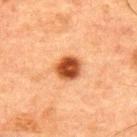notes: total-body-photography surveillance lesion; no biopsy | image source: ~15 mm tile from a whole-body skin photo | illumination: cross-polarized | lesion diameter: ~3.5 mm (longest diameter) | patient: male, aged 48–52 | automated lesion analysis: a mean CIELAB color near L≈42 a*≈25 b*≈35, roughly 16 lightness units darker than nearby skin, and a normalized lesion–skin contrast near 12 | site: the upper back.